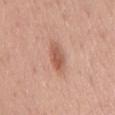Clinical impression: Part of a total-body skin-imaging series; this lesion was reviewed on a skin check and was not flagged for biopsy. Clinical summary: The tile uses white-light illumination. On the chest. This image is a 15 mm lesion crop taken from a total-body photograph. Measured at roughly 4.5 mm in maximum diameter. A male patient roughly 50 years of age.